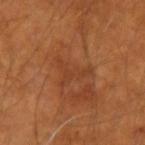Recorded during total-body skin imaging; not selected for excision or biopsy. A male subject, in their 50s. Cropped from a whole-body photographic skin survey; the tile spans about 15 mm. The lesion is on the left forearm.